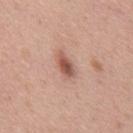No biopsy was performed on this lesion — it was imaged during a full skin examination and was not determined to be concerning. A close-up tile cropped from a whole-body skin photograph, about 15 mm across. The total-body-photography lesion software estimated an eccentricity of roughly 0.85 and a shape-asymmetry score of about 0.25 (0 = symmetric). The analysis additionally found a within-lesion color-variation index near 5/10 and peripheral color asymmetry of about 1.5. The software also gave a nevus-likeness score of about 95/100. The tile uses white-light illumination. About 3 mm across. The subject is a male in their 40s. Located on the mid back.